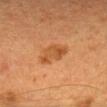The lesion was photographed on a routine skin check and not biopsied; there is no pathology result. A female subject, approximately 40 years of age. Cropped from a whole-body photographic skin survey; the tile spans about 15 mm. The total-body-photography lesion software estimated a footprint of about 8.5 mm² and an eccentricity of roughly 0.75. The software also gave radial color variation of about 1. Measured at roughly 4 mm in maximum diameter. Located on the head or neck. This is a cross-polarized tile.